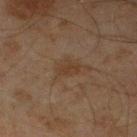tile lighting=cross-polarized illumination | TBP lesion metrics=border irregularity of about 3 on a 0–10 scale, a color-variation rating of about 1.5/10, and a peripheral color-asymmetry measure near 0.5; a nevus-likeness score of about 5/100 and lesion-presence confidence of about 100/100 | patient=male, aged 43 to 47 | image source=total-body-photography crop, ~15 mm field of view | lesion size=≈3 mm | site=the left lower leg.